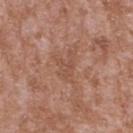patient = male, aged 43–47 | image = ~15 mm tile from a whole-body skin photo | site = the upper back | TBP lesion metrics = a footprint of about 5.5 mm², a shape eccentricity near 0.8, and two-axis asymmetry of about 0.55; a lesion color around L≈51 a*≈21 b*≈29 in CIELAB and a normalized lesion–skin contrast near 4.5; a border-irregularity index near 6.5/10 and peripheral color asymmetry of about 0.5; a nevus-likeness score of about 0/100 | lesion size = ≈3.5 mm.